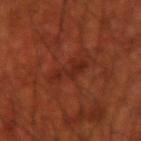notes = catalogued during a skin exam; not biopsied | lighting = cross-polarized illumination | body site = the right upper arm | patient = male, aged around 70 | diameter = ≈4 mm | imaging modality = ~15 mm crop, total-body skin-cancer survey.